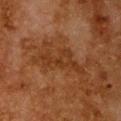Assessment: The lesion was tiled from a total-body skin photograph and was not biopsied. Context: The lesion is on the chest. An algorithmic analysis of the crop reported a lesion color around L≈29 a*≈20 b*≈30 in CIELAB, a lesion–skin lightness drop of about 6, and a normalized lesion–skin contrast near 6.5. The analysis additionally found a border-irregularity index near 9.5/10, a within-lesion color-variation index near 2.5/10, and peripheral color asymmetry of about 0.5. A region of skin cropped from a whole-body photographic capture, roughly 15 mm wide. A female subject, about 50 years old. The tile uses cross-polarized illumination.